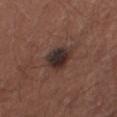Impression: Recorded during total-body skin imaging; not selected for excision or biopsy. Acquisition and patient details: This is a white-light tile. The recorded lesion diameter is about 4 mm. The total-body-photography lesion software estimated border irregularity of about 2 on a 0–10 scale, a color-variation rating of about 6.5/10, and peripheral color asymmetry of about 2. It also reported a lesion-detection confidence of about 100/100. The lesion is on the right thigh. A close-up tile cropped from a whole-body skin photograph, about 15 mm across. A female subject aged approximately 35.